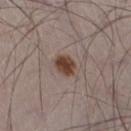* biopsy status — total-body-photography surveillance lesion; no biopsy
* lesion diameter — ≈2.5 mm
* TBP lesion metrics — a lesion area of about 5 mm², a shape eccentricity near 0.6, and a symmetry-axis asymmetry near 0.2; a mean CIELAB color near L≈41 a*≈17 b*≈24, roughly 13 lightness units darker than nearby skin, and a normalized lesion–skin contrast near 11
* lighting — white-light illumination
* subject — male, about 50 years old
* location — the right thigh
* acquisition — ~15 mm tile from a whole-body skin photo